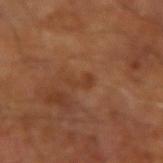{
  "biopsy_status": "not biopsied; imaged during a skin examination",
  "lighting": "cross-polarized",
  "patient": {
    "sex": "male",
    "age_approx": 65
  },
  "image": {
    "source": "total-body photography crop",
    "field_of_view_mm": 15
  }
}